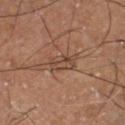The lesion was tiled from a total-body skin photograph and was not biopsied. A male subject aged approximately 60. Approximately 3.5 mm at its widest. The tile uses cross-polarized illumination. Located on the left thigh. This image is a 15 mm lesion crop taken from a total-body photograph.